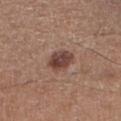Imaged during a routine full-body skin examination; the lesion was not biopsied and no histopathology is available.
A male subject aged approximately 65.
A 15 mm close-up tile from a total-body photography series done for melanoma screening.
From the right lower leg.
Automated image analysis of the tile measured a lesion color around L≈43 a*≈20 b*≈23 in CIELAB, a lesion–skin lightness drop of about 12, and a lesion-to-skin contrast of about 9.5 (normalized; higher = more distinct). The software also gave a border-irregularity index near 2/10, a within-lesion color-variation index near 4/10, and radial color variation of about 1.
The tile uses white-light illumination.